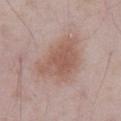The lesion was photographed on a routine skin check and not biopsied; there is no pathology result. From the abdomen. About 6.5 mm across. A male subject, aged around 55. Cropped from a whole-body photographic skin survey; the tile spans about 15 mm. This is a white-light tile.